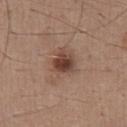body site = the chest
image source = total-body-photography crop, ~15 mm field of view
diameter = ≈4.5 mm
patient = male, aged around 25
tile lighting = white-light illumination
automated metrics = border irregularity of about 3.5 on a 0–10 scale and internal color variation of about 5.5 on a 0–10 scale; an automated nevus-likeness rating near 85 out of 100